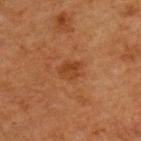notes: imaged on a skin check; not biopsied
image-analysis metrics: border irregularity of about 3 on a 0–10 scale, a color-variation rating of about 2.5/10, and peripheral color asymmetry of about 1
body site: the back
size: ≈2.5 mm
illumination: cross-polarized
image source: 15 mm crop, total-body photography
subject: male, aged 63 to 67